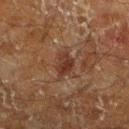Q: Was a biopsy performed?
A: no biopsy performed (imaged during a skin exam)
Q: Patient demographics?
A: male, aged 58 to 62
Q: Lesion location?
A: the left lower leg
Q: What did automated image analysis measure?
A: a classifier nevus-likeness of about 0/100 and a detector confidence of about 95 out of 100 that the crop contains a lesion
Q: What is the lesion's diameter?
A: about 3.5 mm
Q: How was the tile lit?
A: cross-polarized illumination
Q: How was this image acquired?
A: ~15 mm crop, total-body skin-cancer survey